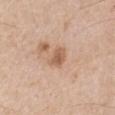{"biopsy_status": "not biopsied; imaged during a skin examination", "patient": {"sex": "male", "age_approx": 65}, "lesion_size": {"long_diameter_mm_approx": 3.0}, "image": {"source": "total-body photography crop", "field_of_view_mm": 15}, "site": "right upper arm"}